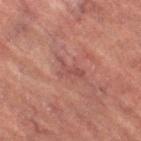On the right thigh.
A 15 mm close-up extracted from a 3D total-body photography capture.
Approximately 3.5 mm at its widest.
This is a cross-polarized tile.
Automated image analysis of the tile measured a nevus-likeness score of about 0/100 and a lesion-detection confidence of about 65/100.
The subject is a female in their 70s.